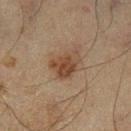Q: Was this lesion biopsied?
A: no biopsy performed (imaged during a skin exam)
Q: Illumination type?
A: cross-polarized
Q: How was this image acquired?
A: ~15 mm crop, total-body skin-cancer survey
Q: What is the anatomic site?
A: the left lower leg
Q: What is the lesion's diameter?
A: ≈4 mm
Q: Who is the patient?
A: male, roughly 65 years of age